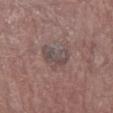Q: Was this lesion biopsied?
A: no biopsy performed (imaged during a skin exam)
Q: What lighting was used for the tile?
A: white-light
Q: Lesion location?
A: the left forearm
Q: How large is the lesion?
A: about 4 mm
Q: What kind of image is this?
A: total-body-photography crop, ~15 mm field of view
Q: Who is the patient?
A: male, in their 80s
Q: Automated lesion metrics?
A: a mean CIELAB color near L≈46 a*≈13 b*≈16, about 7 CIELAB-L* units darker than the surrounding skin, and a lesion-to-skin contrast of about 6 (normalized; higher = more distinct)